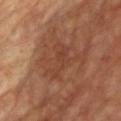  biopsy_status: not biopsied; imaged during a skin examination
  lighting: cross-polarized
  lesion_size:
    long_diameter_mm_approx: 5.0
  site: front of the torso
  patient:
    sex: male
    age_approx: 60
  automated_metrics:
    border_irregularity_0_10: 7.0
    color_variation_0_10: 1.5
    peripheral_color_asymmetry: 0.0
  image:
    source: total-body photography crop
    field_of_view_mm: 15